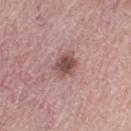location: the left thigh | lighting: white-light | acquisition: total-body-photography crop, ~15 mm field of view | diameter: ≈3 mm | automated metrics: an average lesion color of about L≈49 a*≈22 b*≈20 (CIELAB); a border-irregularity index near 2.5/10, a color-variation rating of about 3.5/10, and peripheral color asymmetry of about 1 | subject: female, aged 63 to 67.